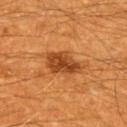The tile uses cross-polarized illumination.
Automated image analysis of the tile measured a lesion color around L≈43 a*≈26 b*≈40 in CIELAB, about 12 CIELAB-L* units darker than the surrounding skin, and a normalized lesion–skin contrast near 9.
Approximately 5 mm at its widest.
A male patient about 60 years old.
From the upper back.
A region of skin cropped from a whole-body photographic capture, roughly 15 mm wide.